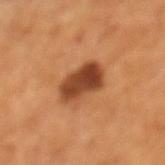Q: Is there a histopathology result?
A: total-body-photography surveillance lesion; no biopsy
Q: What kind of image is this?
A: ~15 mm tile from a whole-body skin photo
Q: How large is the lesion?
A: ~4.5 mm (longest diameter)
Q: What did automated image analysis measure?
A: a mean CIELAB color near L≈43 a*≈26 b*≈36, roughly 15 lightness units darker than nearby skin, and a normalized lesion–skin contrast near 11; border irregularity of about 1.5 on a 0–10 scale, a color-variation rating of about 5.5/10, and peripheral color asymmetry of about 2; a nevus-likeness score of about 70/100
Q: Where on the body is the lesion?
A: the left lower leg
Q: What are the patient's age and sex?
A: female, aged around 65